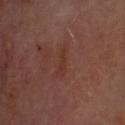notes: imaged on a skin check; not biopsied | patient: approximately 65 years of age | acquisition: ~15 mm crop, total-body skin-cancer survey | lighting: cross-polarized | image-analysis metrics: an outline eccentricity of about 0.95 (0 = round, 1 = elongated) and two-axis asymmetry of about 0.45; a classifier nevus-likeness of about 0/100 and a detector confidence of about 95 out of 100 that the crop contains a lesion | anatomic site: the head or neck | size: ≈3 mm.